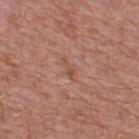Part of a total-body skin-imaging series; this lesion was reviewed on a skin check and was not flagged for biopsy.
The subject is a male roughly 70 years of age.
The lesion's longest dimension is about 3 mm.
From the upper back.
A 15 mm close-up extracted from a 3D total-body photography capture.
Captured under white-light illumination.